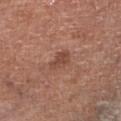Assessment: The lesion was photographed on a routine skin check and not biopsied; there is no pathology result. Background: The lesion is located on the right lower leg. Automated image analysis of the tile measured an average lesion color of about L≈46 a*≈23 b*≈28 (CIELAB), about 9 CIELAB-L* units darker than the surrounding skin, and a lesion-to-skin contrast of about 6.5 (normalized; higher = more distinct). And it measured border irregularity of about 3 on a 0–10 scale and a peripheral color-asymmetry measure near 0.5. This is a white-light tile. A male subject aged 68 to 72. Cropped from a whole-body photographic skin survey; the tile spans about 15 mm. Measured at roughly 3 mm in maximum diameter.